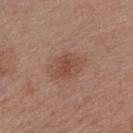Imaged during a routine full-body skin examination; the lesion was not biopsied and no histopathology is available.
A 15 mm close-up extracted from a 3D total-body photography capture.
The tile uses white-light illumination.
The subject is a female aged 53–57.
On the right upper arm.
The recorded lesion diameter is about 4.5 mm.